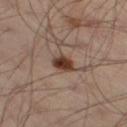<case>
  <biopsy_status>not biopsied; imaged during a skin examination</biopsy_status>
  <image>
    <source>total-body photography crop</source>
    <field_of_view_mm>15</field_of_view_mm>
  </image>
  <lighting>cross-polarized</lighting>
  <site>abdomen</site>
  <lesion_size>
    <long_diameter_mm_approx>3.5</long_diameter_mm_approx>
  </lesion_size>
  <patient>
    <sex>male</sex>
    <age_approx>50</age_approx>
  </patient>
</case>